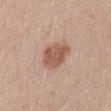notes = no biopsy performed (imaged during a skin exam)
diameter = about 3.5 mm
image = total-body-photography crop, ~15 mm field of view
site = the lower back
automated metrics = a lesion color around L≈55 a*≈20 b*≈28 in CIELAB, a lesion–skin lightness drop of about 11, and a normalized border contrast of about 8; a border-irregularity index near 2/10 and internal color variation of about 2.5 on a 0–10 scale
subject = female, about 55 years old